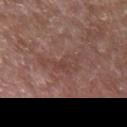* biopsy status: catalogued during a skin exam; not biopsied
* anatomic site: the left forearm
* image: ~15 mm tile from a whole-body skin photo
* illumination: white-light illumination
* lesion diameter: ≈6 mm
* TBP lesion metrics: a border-irregularity rating of about 7.5/10, a within-lesion color-variation index near 2.5/10, and a peripheral color-asymmetry measure near 1
* patient: male, aged approximately 80